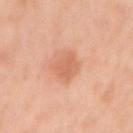Automated image analysis of the tile measured an average lesion color of about L≈65 a*≈26 b*≈35 (CIELAB), roughly 8 lightness units darker than nearby skin, and a normalized border contrast of about 5.5. This is a white-light tile. The recorded lesion diameter is about 3.5 mm. The lesion is on the right upper arm. Cropped from a total-body skin-imaging series; the visible field is about 15 mm. The patient is a female in their mid-40s.